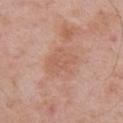notes = total-body-photography surveillance lesion; no biopsy
illumination = white-light illumination
lesion size = ~4 mm (longest diameter)
anatomic site = the arm
patient = male, about 55 years old
imaging modality = 15 mm crop, total-body photography
image-analysis metrics = a border-irregularity index near 3/10 and a color-variation rating of about 2/10; a classifier nevus-likeness of about 0/100 and a detector confidence of about 100 out of 100 that the crop contains a lesion